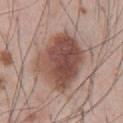biopsy status: total-body-photography surveillance lesion; no biopsy
subject: male, roughly 55 years of age
body site: the abdomen
size: ~6.5 mm (longest diameter)
tile lighting: white-light
image source: ~15 mm tile from a whole-body skin photo